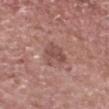biopsy status — imaged on a skin check; not biopsied
size — about 4 mm
lighting — white-light
site — the head or neck
automated lesion analysis — an area of roughly 6.5 mm², a shape eccentricity near 0.75, and a shape-asymmetry score of about 0.4 (0 = symmetric); a lesion color around L≈49 a*≈22 b*≈23 in CIELAB, a lesion–skin lightness drop of about 9, and a lesion-to-skin contrast of about 7 (normalized; higher = more distinct); a nevus-likeness score of about 0/100 and a lesion-detection confidence of about 100/100
subject — male, in their 70s
image source — 15 mm crop, total-body photography